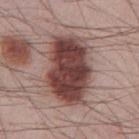follow-up — total-body-photography surveillance lesion; no biopsy
lighting — white-light illumination
lesion diameter — about 7.5 mm
subject — male, in their mid-60s
site — the front of the torso
acquisition — ~15 mm tile from a whole-body skin photo